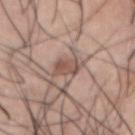| key | value |
|---|---|
| workup | imaged on a skin check; not biopsied |
| image | ~15 mm crop, total-body skin-cancer survey |
| patient | male, aged around 20 |
| illumination | white-light |
| anatomic site | the abdomen |
| TBP lesion metrics | a footprint of about 5 mm², an eccentricity of roughly 0.7, and two-axis asymmetry of about 0.25; a classifier nevus-likeness of about 45/100 |